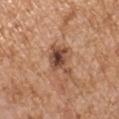<case>
<biopsy_status>not biopsied; imaged during a skin examination</biopsy_status>
<lesion_size>
  <long_diameter_mm_approx>4.0</long_diameter_mm_approx>
</lesion_size>
<site>left upper arm</site>
<lighting>white-light</lighting>
<patient>
  <sex>male</sex>
  <age_approx>65</age_approx>
</patient>
<image>
  <source>total-body photography crop</source>
  <field_of_view_mm>15</field_of_view_mm>
</image>
</case>